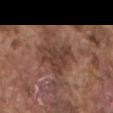Q: Was a biopsy performed?
A: total-body-photography surveillance lesion; no biopsy
Q: How was this image acquired?
A: total-body-photography crop, ~15 mm field of view
Q: What lighting was used for the tile?
A: white-light illumination
Q: Lesion location?
A: the mid back
Q: Patient demographics?
A: male, about 75 years old
Q: Automated lesion metrics?
A: a mean CIELAB color near L≈40 a*≈19 b*≈25, roughly 9 lightness units darker than nearby skin, and a normalized lesion–skin contrast near 7.5; a color-variation rating of about 3/10; an automated nevus-likeness rating near 0 out of 100 and lesion-presence confidence of about 90/100